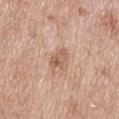Clinical impression:
Part of a total-body skin-imaging series; this lesion was reviewed on a skin check and was not flagged for biopsy.
Clinical summary:
Located on the mid back. A male subject approximately 60 years of age. The lesion's longest dimension is about 3 mm. A 15 mm close-up tile from a total-body photography series done for melanoma screening. This is a white-light tile.